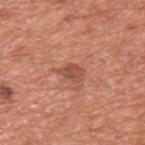Approximately 3 mm at its widest. The lesion-visualizer software estimated a within-lesion color-variation index near 4.5/10 and a peripheral color-asymmetry measure near 1.5. And it measured a nevus-likeness score of about 15/100 and lesion-presence confidence of about 100/100. The patient is a male aged approximately 65. This is a white-light tile. Located on the left upper arm. A lesion tile, about 15 mm wide, cut from a 3D total-body photograph.